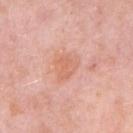– biopsy status · imaged on a skin check; not biopsied
– lesion size · ~3.5 mm (longest diameter)
– acquisition · total-body-photography crop, ~15 mm field of view
– anatomic site · the arm
– TBP lesion metrics · an eccentricity of roughly 0.55; an average lesion color of about L≈66 a*≈25 b*≈32 (CIELAB) and a normalized border contrast of about 5.5; border irregularity of about 3.5 on a 0–10 scale, a within-lesion color-variation index near 2.5/10, and a peripheral color-asymmetry measure near 1
– subject · female, about 45 years old
– lighting · white-light illumination